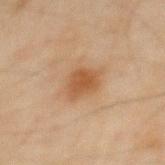notes: catalogued during a skin exam; not biopsied
automated metrics: a footprint of about 7.5 mm², an outline eccentricity of about 0.55 (0 = round, 1 = elongated), and a shape-asymmetry score of about 0.25 (0 = symmetric); an average lesion color of about L≈43 a*≈18 b*≈31 (CIELAB), about 8 CIELAB-L* units darker than the surrounding skin, and a normalized border contrast of about 7.5; a border-irregularity index near 2.5/10, a color-variation rating of about 2/10, and radial color variation of about 0.5
lesion size: about 3.5 mm
image: total-body-photography crop, ~15 mm field of view
illumination: cross-polarized
location: the mid back
subject: male, in their mid- to late 40s